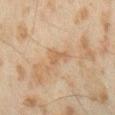Clinical impression: This lesion was catalogued during total-body skin photography and was not selected for biopsy.